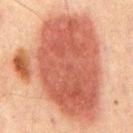workup: catalogued during a skin exam; not biopsied | subject: male, aged 63–67 | lighting: cross-polarized illumination | imaging modality: 15 mm crop, total-body photography | automated lesion analysis: a footprint of about 85 mm², an eccentricity of roughly 0.6, and a shape-asymmetry score of about 0.4 (0 = symmetric); a border-irregularity rating of about 4/10 and peripheral color asymmetry of about 1.5; a detector confidence of about 100 out of 100 that the crop contains a lesion | anatomic site: the back | lesion diameter: about 12.5 mm.